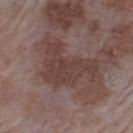follow-up: no biopsy performed (imaged during a skin exam)
patient: male, approximately 65 years of age
image source: ~15 mm tile from a whole-body skin photo
TBP lesion metrics: an area of roughly 21 mm², a shape eccentricity near 0.75, and a symmetry-axis asymmetry near 0.6; a lesion color around L≈40 a*≈18 b*≈21 in CIELAB, about 7 CIELAB-L* units darker than the surrounding skin, and a lesion-to-skin contrast of about 6.5 (normalized; higher = more distinct); a border-irregularity index near 8.5/10 and internal color variation of about 2.5 on a 0–10 scale
lighting: white-light
location: the left upper arm